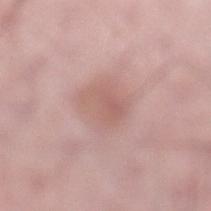{"biopsy_status": "not biopsied; imaged during a skin examination", "lesion_size": {"long_diameter_mm_approx": 4.0}, "image": {"source": "total-body photography crop", "field_of_view_mm": 15}, "site": "left lower leg", "lighting": "white-light", "patient": {"sex": "male", "age_approx": 50}}